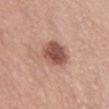  biopsy_status: not biopsied; imaged during a skin examination